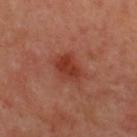Acquisition and patient details:
A region of skin cropped from a whole-body photographic capture, roughly 15 mm wide. The recorded lesion diameter is about 3.5 mm. The lesion is on the back. Captured under cross-polarized illumination. The patient is a male about 50 years old. The total-body-photography lesion software estimated a lesion-detection confidence of about 100/100.